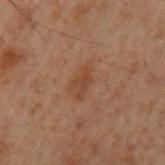notes = catalogued during a skin exam; not biopsied | image source = ~15 mm tile from a whole-body skin photo | size = ≈3 mm | automated metrics = two-axis asymmetry of about 0.55; a border-irregularity rating of about 6.5/10 and radial color variation of about 0; a classifier nevus-likeness of about 15/100 and lesion-presence confidence of about 100/100 | patient = male, about 60 years old | tile lighting = cross-polarized | site = the left upper arm.